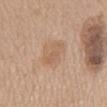<case>
<biopsy_status>not biopsied; imaged during a skin examination</biopsy_status>
<lesion_size>
  <long_diameter_mm_approx>5.0</long_diameter_mm_approx>
</lesion_size>
<site>mid back</site>
<patient>
  <sex>female</sex>
  <age_approx>70</age_approx>
</patient>
<image>
  <source>total-body photography crop</source>
  <field_of_view_mm>15</field_of_view_mm>
</image>
</case>